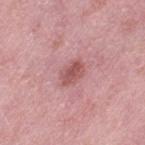{
  "biopsy_status": "not biopsied; imaged during a skin examination"
}